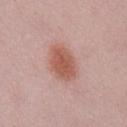| feature | finding |
|---|---|
| follow-up | catalogued during a skin exam; not biopsied |
| image | 15 mm crop, total-body photography |
| subject | female, approximately 25 years of age |
| location | the chest |
| lesion diameter | ≈4.5 mm |
| lighting | white-light |